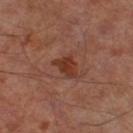The lesion was tiled from a total-body skin photograph and was not biopsied. From the right thigh. About 3 mm across. Automated image analysis of the tile measured a shape-asymmetry score of about 0.45 (0 = symmetric). And it measured about 9 CIELAB-L* units darker than the surrounding skin and a normalized lesion–skin contrast near 8.5. The software also gave border irregularity of about 4 on a 0–10 scale, internal color variation of about 2 on a 0–10 scale, and peripheral color asymmetry of about 0.5. Captured under cross-polarized illumination. A male patient, aged 63 to 67. A region of skin cropped from a whole-body photographic capture, roughly 15 mm wide.